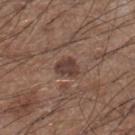No biopsy was performed on this lesion — it was imaged during a full skin examination and was not determined to be concerning.
A male patient about 60 years old.
The lesion-visualizer software estimated a footprint of about 5 mm², a shape eccentricity near 0.5, and two-axis asymmetry of about 0.3. The software also gave a lesion color around L≈39 a*≈18 b*≈22 in CIELAB and a normalized lesion–skin contrast near 8.5. And it measured a nevus-likeness score of about 35/100 and a detector confidence of about 95 out of 100 that the crop contains a lesion.
This is a white-light tile.
Longest diameter approximately 3 mm.
A roughly 15 mm field-of-view crop from a total-body skin photograph.
Located on the right lower leg.